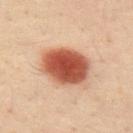Background: A roughly 15 mm field-of-view crop from a total-body skin photograph. The lesion is on the mid back. The patient is a male aged approximately 50.